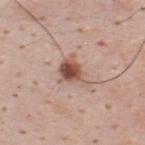Case summary:
* notes — no biopsy performed (imaged during a skin exam)
* subject — male, aged around 35
* illumination — white-light illumination
* image — ~15 mm crop, total-body skin-cancer survey
* automated lesion analysis — a lesion area of about 7.5 mm², an outline eccentricity of about 0.65 (0 = round, 1 = elongated), and two-axis asymmetry of about 0.4; an average lesion color of about L≈53 a*≈20 b*≈26 (CIELAB) and a normalized border contrast of about 9; a border-irregularity rating of about 5/10, a within-lesion color-variation index near 7.5/10, and peripheral color asymmetry of about 3
* location — the upper back